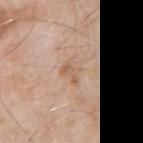{
  "biopsy_status": "not biopsied; imaged during a skin examination",
  "image": {
    "source": "total-body photography crop",
    "field_of_view_mm": 15
  },
  "site": "left upper arm",
  "lesion_size": {
    "long_diameter_mm_approx": 3.0
  },
  "lighting": "white-light",
  "patient": {
    "sex": "male",
    "age_approx": 55
  }
}